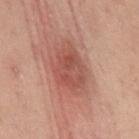notes — catalogued during a skin exam; not biopsied
site — the mid back
diameter — ≈6 mm
tile lighting — cross-polarized illumination
acquisition — 15 mm crop, total-body photography
patient — female, in their mid- to late 50s